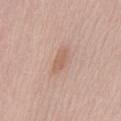A female patient aged approximately 30.
Cropped from a whole-body photographic skin survey; the tile spans about 15 mm.
Located on the mid back.